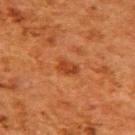Q: Is there a histopathology result?
A: catalogued during a skin exam; not biopsied
Q: What is the imaging modality?
A: 15 mm crop, total-body photography
Q: Lesion size?
A: about 2.5 mm
Q: Illumination type?
A: cross-polarized
Q: What did automated image analysis measure?
A: a mean CIELAB color near L≈36 a*≈27 b*≈35, a lesion–skin lightness drop of about 8, and a normalized border contrast of about 7; a border-irregularity rating of about 3/10, a within-lesion color-variation index near 2/10, and radial color variation of about 1
Q: What is the anatomic site?
A: the upper back
Q: What are the patient's age and sex?
A: female, aged approximately 50